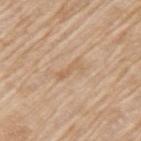Imaged during a routine full-body skin examination; the lesion was not biopsied and no histopathology is available.
Approximately 3 mm at its widest.
Imaged with white-light lighting.
The total-body-photography lesion software estimated a mean CIELAB color near L≈62 a*≈17 b*≈34, roughly 6 lightness units darker than nearby skin, and a normalized lesion–skin contrast near 5. The analysis additionally found internal color variation of about 0 on a 0–10 scale. And it measured an automated nevus-likeness rating near 0 out of 100 and a lesion-detection confidence of about 85/100.
A female subject aged around 75.
From the left upper arm.
A close-up tile cropped from a whole-body skin photograph, about 15 mm across.